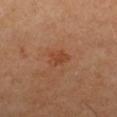patient: female, about 50 years old
diameter: ≈3 mm
image-analysis metrics: an area of roughly 4.5 mm², a shape eccentricity near 0.65, and a shape-asymmetry score of about 0.3 (0 = symmetric); an average lesion color of about L≈44 a*≈25 b*≈33 (CIELAB), a lesion–skin lightness drop of about 7, and a normalized border contrast of about 5.5; a border-irregularity index near 3/10
image: ~15 mm crop, total-body skin-cancer survey